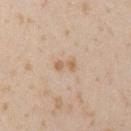notes: imaged on a skin check; not biopsied | illumination: white-light | anatomic site: the left upper arm | imaging modality: ~15 mm crop, total-body skin-cancer survey | image-analysis metrics: a mean CIELAB color near L≈63 a*≈16 b*≈32 and a normalized lesion–skin contrast near 6.5; a classifier nevus-likeness of about 0/100 and a lesion-detection confidence of about 100/100 | subject: male, aged 23 to 27 | lesion size: ~2.5 mm (longest diameter).